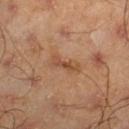Case summary:
• size: ~4.5 mm (longest diameter)
• automated lesion analysis: a footprint of about 6.5 mm² and a symmetry-axis asymmetry near 0.25; a border-irregularity index near 3.5/10, internal color variation of about 5 on a 0–10 scale, and radial color variation of about 1.5; a nevus-likeness score of about 0/100 and lesion-presence confidence of about 90/100
• body site: the right lower leg
• imaging modality: total-body-photography crop, ~15 mm field of view
• subject: male, aged approximately 45